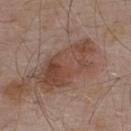The lesion was tiled from a total-body skin photograph and was not biopsied.
The lesion's longest dimension is about 7.5 mm.
A 15 mm close-up tile from a total-body photography series done for melanoma screening.
Located on the upper back.
A male subject aged 53–57.